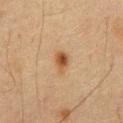This lesion was catalogued during total-body skin photography and was not selected for biopsy. Cropped from a total-body skin-imaging series; the visible field is about 15 mm. On the abdomen. A male subject in their 60s. Automated image analysis of the tile measured a lesion area of about 4.5 mm², an eccentricity of roughly 0.8, and a symmetry-axis asymmetry near 0.2. It also reported a mean CIELAB color near L≈41 a*≈16 b*≈30 and a normalized border contrast of about 8. The analysis additionally found a border-irregularity rating of about 2/10 and radial color variation of about 1.5. The software also gave a nevus-likeness score of about 95/100 and a lesion-detection confidence of about 100/100.